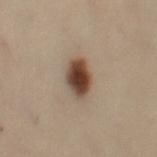Q: Was this lesion biopsied?
A: imaged on a skin check; not biopsied
Q: Who is the patient?
A: female, aged around 50
Q: How was the tile lit?
A: cross-polarized illumination
Q: How was this image acquired?
A: ~15 mm crop, total-body skin-cancer survey
Q: What is the lesion's diameter?
A: ≈3.5 mm
Q: What is the anatomic site?
A: the right thigh